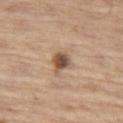Impression: Recorded during total-body skin imaging; not selected for excision or biopsy. Context: Located on the left thigh. A male subject about 70 years old. The tile uses white-light illumination. Measured at roughly 2.5 mm in maximum diameter. Automated image analysis of the tile measured an area of roughly 5 mm², a shape eccentricity near 0.4, and a shape-asymmetry score of about 0.25 (0 = symmetric). It also reported an average lesion color of about L≈52 a*≈17 b*≈30 (CIELAB), about 14 CIELAB-L* units darker than the surrounding skin, and a normalized border contrast of about 9.5. It also reported a border-irregularity index near 2.5/10, a within-lesion color-variation index near 6/10, and radial color variation of about 2. The software also gave an automated nevus-likeness rating near 90 out of 100. A roughly 15 mm field-of-view crop from a total-body skin photograph.